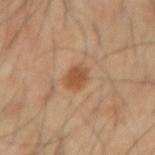Assessment:
Imaged during a routine full-body skin examination; the lesion was not biopsied and no histopathology is available.
Image and clinical context:
The lesion is on the right forearm. The subject is a male aged 53 to 57. Captured under cross-polarized illumination. The total-body-photography lesion software estimated a classifier nevus-likeness of about 90/100 and lesion-presence confidence of about 100/100. The recorded lesion diameter is about 2.5 mm. A region of skin cropped from a whole-body photographic capture, roughly 15 mm wide.